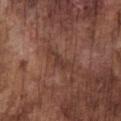Impression: No biopsy was performed on this lesion — it was imaged during a full skin examination and was not determined to be concerning. Clinical summary: A male patient, in their mid-70s. A 15 mm close-up tile from a total-body photography series done for melanoma screening. On the chest.